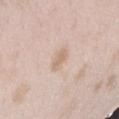- workup · total-body-photography surveillance lesion; no biopsy
- image · total-body-photography crop, ~15 mm field of view
- illumination · white-light
- subject · female, approximately 25 years of age
- body site · the left forearm
- lesion size · ≈2.5 mm